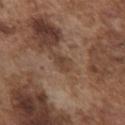No biopsy was performed on this lesion — it was imaged during a full skin examination and was not determined to be concerning. About 3 mm across. A male subject, in their mid-70s. A close-up tile cropped from a whole-body skin photograph, about 15 mm across. From the chest. This is a white-light tile.